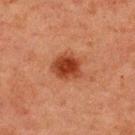No biopsy was performed on this lesion — it was imaged during a full skin examination and was not determined to be concerning.
A 15 mm close-up extracted from a 3D total-body photography capture.
Approximately 3.5 mm at its widest.
Located on the upper back.
Captured under cross-polarized illumination.
A male subject, aged 58–62.